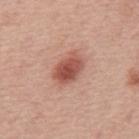• workup — imaged on a skin check; not biopsied
• acquisition — 15 mm crop, total-body photography
• lighting — white-light
• anatomic site — the mid back
• patient — male, aged approximately 75